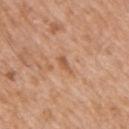Imaged during a routine full-body skin examination; the lesion was not biopsied and no histopathology is available. The patient is a male in their mid- to late 70s. About 2.5 mm across. From the arm. This is a white-light tile. An algorithmic analysis of the crop reported an area of roughly 2.5 mm² and an eccentricity of roughly 0.9. The software also gave an average lesion color of about L≈57 a*≈23 b*≈35 (CIELAB) and about 9 CIELAB-L* units darker than the surrounding skin. The analysis additionally found a within-lesion color-variation index near 0/10 and radial color variation of about 0. A 15 mm close-up extracted from a 3D total-body photography capture.